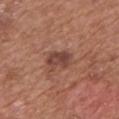| feature | finding |
|---|---|
| notes | no biopsy performed (imaged during a skin exam) |
| acquisition | 15 mm crop, total-body photography |
| patient | male, aged 73 to 77 |
| site | the chest |